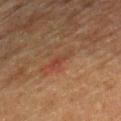notes: no biopsy performed (imaged during a skin exam) | lesion diameter: ≈3 mm | illumination: cross-polarized | site: the right lower leg | imaging modality: total-body-photography crop, ~15 mm field of view | patient: male, aged around 85 or older | TBP lesion metrics: a lesion color around L≈37 a*≈21 b*≈28 in CIELAB, a lesion–skin lightness drop of about 6, and a normalized lesion–skin contrast near 5.5; a border-irregularity rating of about 4.5/10, internal color variation of about 0.5 on a 0–10 scale, and peripheral color asymmetry of about 0.